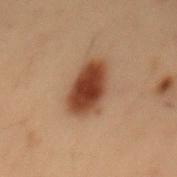Assessment: Captured during whole-body skin photography for melanoma surveillance; the lesion was not biopsied. Image and clinical context: Captured under cross-polarized illumination. A 15 mm close-up tile from a total-body photography series done for melanoma screening. The patient is a male aged 53 to 57. From the mid back. The lesion-visualizer software estimated a lesion area of about 14 mm² and two-axis asymmetry of about 0.15. It also reported an average lesion color of about L≈35 a*≈19 b*≈27 (CIELAB), roughly 14 lightness units darker than nearby skin, and a lesion-to-skin contrast of about 12 (normalized; higher = more distinct). It also reported border irregularity of about 2.5 on a 0–10 scale and a peripheral color-asymmetry measure near 1. And it measured a classifier nevus-likeness of about 100/100. Measured at roughly 6 mm in maximum diameter.